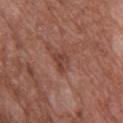Case summary:
• follow-up: imaged on a skin check; not biopsied
• location: the upper back
• subject: female, aged 73–77
• acquisition: ~15 mm crop, total-body skin-cancer survey